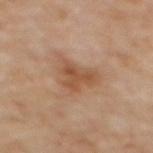Context: A 15 mm close-up extracted from a 3D total-body photography capture. Automated tile analysis of the lesion measured border irregularity of about 5 on a 0–10 scale, a within-lesion color-variation index near 2.5/10, and peripheral color asymmetry of about 1. A female patient approximately 60 years of age. Measured at roughly 4.5 mm in maximum diameter. From the upper back. Captured under cross-polarized illumination.